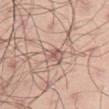<case>
<biopsy_status>not biopsied; imaged during a skin examination</biopsy_status>
<automated_metrics>
  <area_mm2_approx>4.5</area_mm2_approx>
  <shape_asymmetry>0.4</shape_asymmetry>
  <vs_skin_darker_L>9.0</vs_skin_darker_L>
  <lesion_detection_confidence_0_100>70</lesion_detection_confidence_0_100>
</automated_metrics>
<lesion_size>
  <long_diameter_mm_approx>3.5</long_diameter_mm_approx>
</lesion_size>
<image>
  <source>total-body photography crop</source>
  <field_of_view_mm>15</field_of_view_mm>
</image>
<patient>
  <sex>male</sex>
  <age_approx>45</age_approx>
</patient>
<lighting>white-light</lighting>
<site>left thigh</site>
</case>